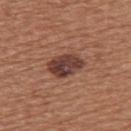Findings:
- workup — catalogued during a skin exam; not biopsied
- anatomic site — the upper back
- subject — female, roughly 55 years of age
- acquisition — 15 mm crop, total-body photography